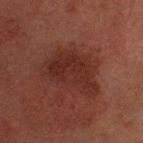Q: Is there a histopathology result?
A: imaged on a skin check; not biopsied
Q: What is the anatomic site?
A: the head or neck
Q: Illumination type?
A: cross-polarized
Q: What kind of image is this?
A: 15 mm crop, total-body photography
Q: What are the patient's age and sex?
A: male, aged approximately 60
Q: What is the lesion's diameter?
A: ~6.5 mm (longest diameter)